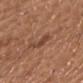biopsy status = total-body-photography surveillance lesion; no biopsy
imaging modality = ~15 mm crop, total-body skin-cancer survey
illumination = white-light
subject = male, aged approximately 70
location = the front of the torso
automated metrics = a lesion-detection confidence of about 100/100
lesion diameter = ≈3 mm